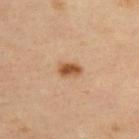Recorded during total-body skin imaging; not selected for excision or biopsy. This is a cross-polarized tile. The lesion is located on the upper back. This image is a 15 mm lesion crop taken from a total-body photograph. Approximately 3 mm at its widest. A male patient, aged around 35.